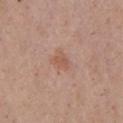<case>
<biopsy_status>not biopsied; imaged during a skin examination</biopsy_status>
<image>
  <source>total-body photography crop</source>
  <field_of_view_mm>15</field_of_view_mm>
</image>
<lesion_size>
  <long_diameter_mm_approx>2.5</long_diameter_mm_approx>
</lesion_size>
<lighting>white-light</lighting>
<automated_metrics>
  <cielab_L>56</cielab_L>
  <cielab_a>22</cielab_a>
  <cielab_b>28</cielab_b>
  <vs_skin_darker_L>7.0</vs_skin_darker_L>
  <vs_skin_contrast_norm>5.5</vs_skin_contrast_norm>
  <border_irregularity_0_10>2.5</border_irregularity_0_10>
  <peripheral_color_asymmetry>0.5</peripheral_color_asymmetry>
</automated_metrics>
<patient>
  <sex>male</sex>
  <age_approx>55</age_approx>
</patient>
<site>chest</site>
</case>